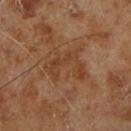follow-up=catalogued during a skin exam; not biopsied | lesion diameter=≈5 mm | patient=male, aged 68 to 72 | anatomic site=the right lower leg | imaging modality=~15 mm crop, total-body skin-cancer survey | automated lesion analysis=a shape eccentricity near 0.8 and two-axis asymmetry of about 0.6 | tile lighting=cross-polarized.